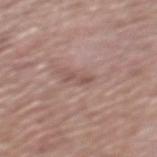workup = imaged on a skin check; not biopsied
illumination = white-light illumination
imaging modality = 15 mm crop, total-body photography
subject = male, in their mid- to late 70s
site = the mid back
size = ≈2.5 mm